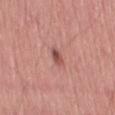Q: Was this lesion biopsied?
A: no biopsy performed (imaged during a skin exam)
Q: What are the patient's age and sex?
A: male, about 80 years old
Q: What is the lesion's diameter?
A: ≈3 mm
Q: Illumination type?
A: white-light illumination
Q: How was this image acquired?
A: ~15 mm tile from a whole-body skin photo
Q: What is the anatomic site?
A: the left thigh